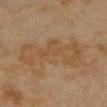This image is a 15 mm lesion crop taken from a total-body photograph. A female subject roughly 35 years of age. About 9 mm across. The lesion-visualizer software estimated a lesion–skin lightness drop of about 6 and a lesion-to-skin contrast of about 5 (normalized; higher = more distinct). The software also gave a nevus-likeness score of about 0/100. This is a cross-polarized tile. From the chest.